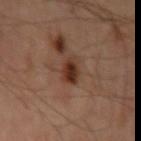Impression: Part of a total-body skin-imaging series; this lesion was reviewed on a skin check and was not flagged for biopsy. Acquisition and patient details: The lesion is located on the left upper arm. A male patient, aged approximately 65. Cropped from a whole-body photographic skin survey; the tile spans about 15 mm.